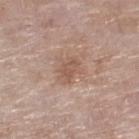Findings:
– biopsy status — total-body-photography surveillance lesion; no biopsy
– illumination — white-light illumination
– imaging modality — ~15 mm tile from a whole-body skin photo
– lesion diameter — ~3 mm (longest diameter)
– patient — female, aged approximately 75
– location — the right lower leg